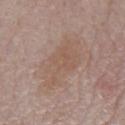No biopsy was performed on this lesion — it was imaged during a full skin examination and was not determined to be concerning. Measured at roughly 7.5 mm in maximum diameter. A 15 mm close-up extracted from a 3D total-body photography capture. The tile uses white-light illumination. Automated image analysis of the tile measured a lesion area of about 18 mm² and a shape eccentricity near 0.9. It also reported a mean CIELAB color near L≈55 a*≈16 b*≈26 and about 5 CIELAB-L* units darker than the surrounding skin. A male subject aged around 75. From the mid back.